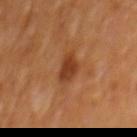Notes:
* biopsy status: imaged on a skin check; not biopsied
* lighting: cross-polarized illumination
* acquisition: ~15 mm tile from a whole-body skin photo
* patient: male, aged around 65
* body site: the mid back
* TBP lesion metrics: an area of roughly 6 mm², an eccentricity of roughly 0.65, and two-axis asymmetry of about 0.25; a mean CIELAB color near L≈38 a*≈25 b*≈34; a border-irregularity rating of about 2.5/10, a color-variation rating of about 2.5/10, and a peripheral color-asymmetry measure near 1; a nevus-likeness score of about 80/100